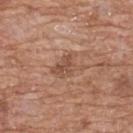Assessment:
The lesion was photographed on a routine skin check and not biopsied; there is no pathology result.
Acquisition and patient details:
The patient is a male aged around 60. From the front of the torso. The tile uses white-light illumination. Measured at roughly 3.5 mm in maximum diameter. Cropped from a total-body skin-imaging series; the visible field is about 15 mm. The lesion-visualizer software estimated a mean CIELAB color near L≈51 a*≈21 b*≈29 and a normalized lesion–skin contrast near 6. The analysis additionally found border irregularity of about 4.5 on a 0–10 scale, a within-lesion color-variation index near 3/10, and peripheral color asymmetry of about 1. It also reported an automated nevus-likeness rating near 0 out of 100 and lesion-presence confidence of about 100/100.